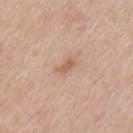{"site": "arm", "automated_metrics": {"area_mm2_approx": 3.0, "eccentricity": 0.85, "shape_asymmetry": 0.4, "vs_skin_contrast_norm": 6.5, "border_irregularity_0_10": 3.5, "color_variation_0_10": 1.0, "peripheral_color_asymmetry": 0.5}, "patient": {"sex": "female", "age_approx": 40}, "image": {"source": "total-body photography crop", "field_of_view_mm": 15}}